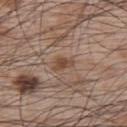workup — total-body-photography surveillance lesion; no biopsy
acquisition — ~15 mm tile from a whole-body skin photo
body site — the upper back
lighting — white-light
subject — male, in their mid- to late 60s
size — ~3 mm (longest diameter)